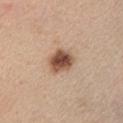Q: Was this lesion biopsied?
A: no biopsy performed (imaged during a skin exam)
Q: Where on the body is the lesion?
A: the chest
Q: What kind of image is this?
A: 15 mm crop, total-body photography
Q: Lesion size?
A: about 3.5 mm
Q: Patient demographics?
A: female, aged 43–47
Q: How was the tile lit?
A: white-light